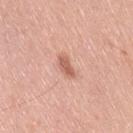biopsy_status: not biopsied; imaged during a skin examination
image:
  source: total-body photography crop
  field_of_view_mm: 15
patient:
  sex: female
  age_approx: 40
site: back
automated_metrics:
  area_mm2_approx: 4.5
  eccentricity: 0.85
  shape_asymmetry: 0.25
  cielab_L: 62
  cielab_a: 24
  cielab_b: 29
  vs_skin_darker_L: 10.0
  vs_skin_contrast_norm: 6.5
  color_variation_0_10: 2.0
  peripheral_color_asymmetry: 0.5